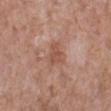Q: Was a biopsy performed?
A: catalogued during a skin exam; not biopsied
Q: What is the imaging modality?
A: ~15 mm crop, total-body skin-cancer survey
Q: Where on the body is the lesion?
A: the left lower leg
Q: Patient demographics?
A: female, in their mid- to late 70s
Q: How large is the lesion?
A: ≈2.5 mm